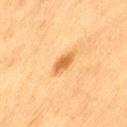Imaged during a routine full-body skin examination; the lesion was not biopsied and no histopathology is available. The patient is a female aged around 55. The lesion is on the left thigh. An algorithmic analysis of the crop reported a lesion area of about 4 mm² and a shape eccentricity near 0.8. And it measured a lesion–skin lightness drop of about 11 and a lesion-to-skin contrast of about 8 (normalized; higher = more distinct). It also reported a nevus-likeness score of about 90/100 and lesion-presence confidence of about 100/100. A 15 mm crop from a total-body photograph taken for skin-cancer surveillance. Longest diameter approximately 3 mm.